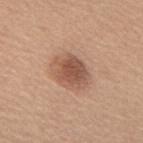Captured during whole-body skin photography for melanoma surveillance; the lesion was not biopsied. Located on the upper back. Cropped from a whole-body photographic skin survey; the tile spans about 15 mm. Measured at roughly 4.5 mm in maximum diameter. The total-body-photography lesion software estimated a footprint of about 11 mm², a shape eccentricity near 0.65, and a symmetry-axis asymmetry near 0.15. And it measured an automated nevus-likeness rating near 85 out of 100. This is a white-light tile. A female subject, aged 63–67.